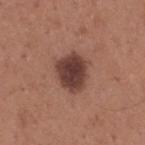This lesion was catalogued during total-body skin photography and was not selected for biopsy. A male patient, roughly 65 years of age. This image is a 15 mm lesion crop taken from a total-body photograph. From the upper back. Measured at roughly 4.5 mm in maximum diameter. Imaged with white-light lighting.